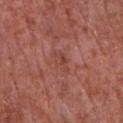Recorded during total-body skin imaging; not selected for excision or biopsy.
Automated image analysis of the tile measured a border-irregularity rating of about 4/10, internal color variation of about 3.5 on a 0–10 scale, and peripheral color asymmetry of about 1.
Captured under white-light illumination.
Located on the chest.
The subject is a male about 65 years old.
The recorded lesion diameter is about 3 mm.
A region of skin cropped from a whole-body photographic capture, roughly 15 mm wide.